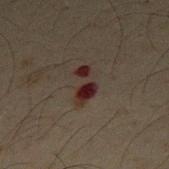Recorded during total-body skin imaging; not selected for excision or biopsy. Located on the chest. A male subject approximately 65 years of age. This image is a 15 mm lesion crop taken from a total-body photograph.